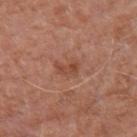| field | value |
|---|---|
| workup | no biopsy performed (imaged during a skin exam) |
| image | ~15 mm tile from a whole-body skin photo |
| diameter | ~2.5 mm (longest diameter) |
| site | the left upper arm |
| tile lighting | white-light |
| subject | male, aged 63 to 67 |